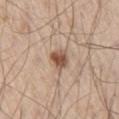Impression:
No biopsy was performed on this lesion — it was imaged during a full skin examination and was not determined to be concerning.
Image and clinical context:
This is a white-light tile. The total-body-photography lesion software estimated an area of roughly 5 mm² and a symmetry-axis asymmetry near 0.25. The software also gave an average lesion color of about L≈53 a*≈19 b*≈29 (CIELAB), a lesion–skin lightness drop of about 14, and a normalized border contrast of about 9.5. The analysis additionally found a border-irregularity index near 2/10 and peripheral color asymmetry of about 1. The patient is a male in their 60s. A lesion tile, about 15 mm wide, cut from a 3D total-body photograph. The lesion is on the right thigh. Longest diameter approximately 2.5 mm.